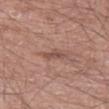Captured during whole-body skin photography for melanoma surveillance; the lesion was not biopsied.
A 15 mm close-up extracted from a 3D total-body photography capture.
A male subject aged 68–72.
The recorded lesion diameter is about 3 mm.
Imaged with white-light lighting.
The lesion is located on the leg.